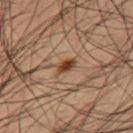Acquisition and patient details: A male patient, in their 40s. A 15 mm crop from a total-body photograph taken for skin-cancer surveillance. The lesion is on the right thigh. About 2.5 mm across. Captured under cross-polarized illumination.